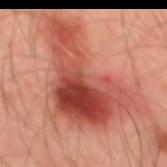workup: catalogued during a skin exam; not biopsied
imaging modality: ~15 mm tile from a whole-body skin photo
automated metrics: a nevus-likeness score of about 5/100 and lesion-presence confidence of about 100/100
illumination: cross-polarized illumination
subject: male, in their mid- to late 40s
site: the mid back